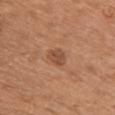A 15 mm close-up tile from a total-body photography series done for melanoma screening.
On the chest.
The patient is a female aged around 65.
The total-body-photography lesion software estimated a mean CIELAB color near L≈49 a*≈23 b*≈31 and roughly 9 lightness units darker than nearby skin. The software also gave a within-lesion color-variation index near 2.5/10 and a peripheral color-asymmetry measure near 1. The analysis additionally found an automated nevus-likeness rating near 40 out of 100 and a lesion-detection confidence of about 100/100.